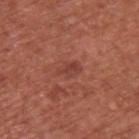biopsy status = catalogued during a skin exam; not biopsied | body site = the back | acquisition = ~15 mm crop, total-body skin-cancer survey | patient = male, aged around 65 | lighting = white-light illumination | diameter = ≈3 mm.